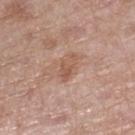Clinical impression: Recorded during total-body skin imaging; not selected for excision or biopsy. Image and clinical context: Cropped from a total-body skin-imaging series; the visible field is about 15 mm. Measured at roughly 3.5 mm in maximum diameter. The tile uses white-light illumination. The lesion is located on the right lower leg. A female subject roughly 70 years of age.